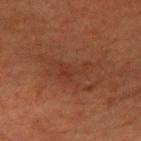  biopsy_status: not biopsied; imaged during a skin examination
  lesion_size:
    long_diameter_mm_approx: 5.5
  lighting: cross-polarized
  patient:
    sex: male
    age_approx: 65
  image:
    source: total-body photography crop
    field_of_view_mm: 15
  site: left upper arm